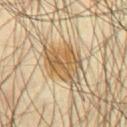A 15 mm crop from a total-body photograph taken for skin-cancer surveillance. The lesion is located on the abdomen. The recorded lesion diameter is about 5 mm. A male patient aged 63 to 67.